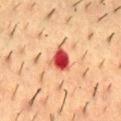Captured during whole-body skin photography for melanoma surveillance; the lesion was not biopsied. On the mid back. A male patient, aged 53 to 57. A 15 mm crop from a total-body photograph taken for skin-cancer surveillance. The tile uses cross-polarized illumination. The recorded lesion diameter is about 3.5 mm.